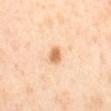{
  "biopsy_status": "not biopsied; imaged during a skin examination",
  "site": "mid back",
  "automated_metrics": {
    "eccentricity": 0.65,
    "cielab_L": 69,
    "cielab_a": 22,
    "cielab_b": 40,
    "vs_skin_darker_L": 13.0,
    "vs_skin_contrast_norm": 8.5,
    "border_irregularity_0_10": 1.5,
    "color_variation_0_10": 3.0,
    "peripheral_color_asymmetry": 1.0,
    "nevus_likeness_0_100": 95,
    "lesion_detection_confidence_0_100": 100
  },
  "patient": {
    "sex": "female",
    "age_approx": 40
  },
  "lighting": "cross-polarized",
  "image": {
    "source": "total-body photography crop",
    "field_of_view_mm": 15
  }
}